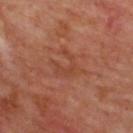image=~15 mm crop, total-body skin-cancer survey
tile lighting=cross-polarized illumination
subject=male, roughly 65 years of age
automated lesion analysis=an eccentricity of roughly 0.6; a lesion–skin lightness drop of about 5 and a normalized border contrast of about 4.5; an automated nevus-likeness rating near 0 out of 100 and a detector confidence of about 100 out of 100 that the crop contains a lesion
lesion diameter=~4.5 mm (longest diameter)
location=the upper back